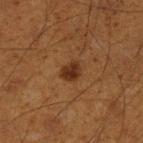patient: male, aged around 60 | image: ~15 mm tile from a whole-body skin photo | lesion diameter: ≈2.5 mm | tile lighting: cross-polarized illumination | body site: the left lower leg | automated metrics: a lesion area of about 4.5 mm² and an eccentricity of roughly 0.6; a lesion color around L≈26 a*≈18 b*≈27 in CIELAB, a lesion–skin lightness drop of about 9, and a lesion-to-skin contrast of about 9.5 (normalized; higher = more distinct).